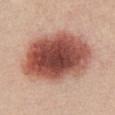workup = no biopsy performed (imaged during a skin exam)
diameter = about 10 mm
subject = female, aged 38–42
illumination = white-light illumination
image source = ~15 mm crop, total-body skin-cancer survey
site = the chest